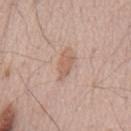  site: mid back
  image:
    source: total-body photography crop
    field_of_view_mm: 15
  lesion_size:
    long_diameter_mm_approx: 4.0
  patient:
    sex: male
    age_approx: 65
  lighting: white-light
  automated_metrics:
    vs_skin_darker_L: 8.0
    vs_skin_contrast_norm: 6.0
    nevus_likeness_0_100: 0
    lesion_detection_confidence_0_100: 100